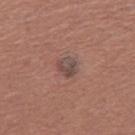| feature | finding |
|---|---|
| biopsy status | total-body-photography surveillance lesion; no biopsy |
| imaging modality | total-body-photography crop, ~15 mm field of view |
| automated metrics | a lesion area of about 4.5 mm², an outline eccentricity of about 0.5 (0 = round, 1 = elongated), and two-axis asymmetry of about 0.1; an automated nevus-likeness rating near 50 out of 100 and a detector confidence of about 100 out of 100 that the crop contains a lesion |
| subject | female, in their 50s |
| location | the right thigh |